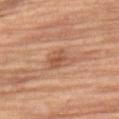No biopsy was performed on this lesion — it was imaged during a full skin examination and was not determined to be concerning. A roughly 15 mm field-of-view crop from a total-body skin photograph. From the right thigh. The subject is a female aged around 70.